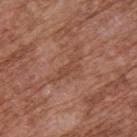Located on the upper back. Captured under white-light illumination. A 15 mm close-up extracted from a 3D total-body photography capture. The lesion-visualizer software estimated an average lesion color of about L≈46 a*≈23 b*≈30 (CIELAB), roughly 6 lightness units darker than nearby skin, and a normalized border contrast of about 5.5. And it measured a border-irregularity index near 7/10, a color-variation rating of about 1/10, and radial color variation of about 0.5. A male subject approximately 75 years of age. Approximately 4 mm at its widest.